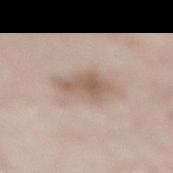Clinical impression: The lesion was photographed on a routine skin check and not biopsied; there is no pathology result. Image and clinical context: Automated image analysis of the tile measured an area of roughly 11 mm² and a shape eccentricity near 0.8. And it measured an average lesion color of about L≈59 a*≈14 b*≈25 (CIELAB) and a normalized border contrast of about 7. It also reported a nevus-likeness score of about 10/100 and a detector confidence of about 100 out of 100 that the crop contains a lesion. A male patient in their 80s. Located on the right lower leg. Measured at roughly 5 mm in maximum diameter. The tile uses white-light illumination. A lesion tile, about 15 mm wide, cut from a 3D total-body photograph.